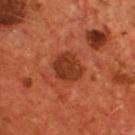follow-up — catalogued during a skin exam; not biopsied
subject — male, aged around 55
body site — the chest
imaging modality — ~15 mm tile from a whole-body skin photo